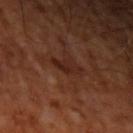Case summary:
* follow-up: total-body-photography surveillance lesion; no biopsy
* lighting: cross-polarized
* location: the left upper arm
* image source: total-body-photography crop, ~15 mm field of view
* subject: in their mid- to late 60s